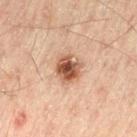Case summary:
* follow-up: total-body-photography surveillance lesion; no biopsy
* size: about 3.5 mm
* illumination: cross-polarized
* patient: male, approximately 45 years of age
* body site: the left thigh
* imaging modality: 15 mm crop, total-body photography
* TBP lesion metrics: an area of roughly 7.5 mm², an eccentricity of roughly 0.25, and two-axis asymmetry of about 0.2; an average lesion color of about L≈47 a*≈20 b*≈28 (CIELAB)